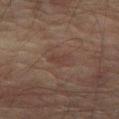The lesion was tiled from a total-body skin photograph and was not biopsied. A region of skin cropped from a whole-body photographic capture, roughly 15 mm wide. The lesion is located on the right thigh. About 2.5 mm across. The lesion-visualizer software estimated a lesion area of about 3 mm² and a shape eccentricity near 0.8. And it measured a border-irregularity rating of about 3.5/10 and radial color variation of about 0. A male patient, approximately 75 years of age. Imaged with cross-polarized lighting.